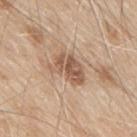Captured during whole-body skin photography for melanoma surveillance; the lesion was not biopsied. On the upper back. The subject is a male about 80 years old. A region of skin cropped from a whole-body photographic capture, roughly 15 mm wide.